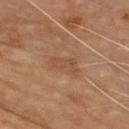notes: imaged on a skin check; not biopsied | image-analysis metrics: a mean CIELAB color near L≈46 a*≈20 b*≈31, roughly 6 lightness units darker than nearby skin, and a lesion-to-skin contrast of about 4.5 (normalized; higher = more distinct); a border-irregularity rating of about 5/10 and peripheral color asymmetry of about 0.5; a nevus-likeness score of about 0/100 | patient: male, in their mid-60s | size: about 3.5 mm | location: the chest | image source: ~15 mm tile from a whole-body skin photo | lighting: cross-polarized illumination.